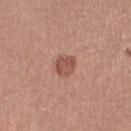The lesion was photographed on a routine skin check and not biopsied; there is no pathology result.
The subject is a female aged approximately 55.
The recorded lesion diameter is about 3 mm.
A 15 mm close-up tile from a total-body photography series done for melanoma screening.
This is a white-light tile.
On the left thigh.